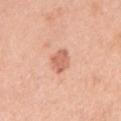The lesion was photographed on a routine skin check and not biopsied; there is no pathology result.
From the right upper arm.
This is a white-light tile.
Automated image analysis of the tile measured a mean CIELAB color near L≈64 a*≈26 b*≈32 and a lesion-to-skin contrast of about 7 (normalized; higher = more distinct).
A 15 mm close-up tile from a total-body photography series done for melanoma screening.
A female patient, aged 58–62.
Approximately 2.5 mm at its widest.